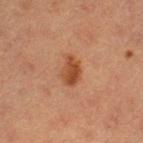<record>
<biopsy_status>not biopsied; imaged during a skin examination</biopsy_status>
<patient>
  <sex>female</sex>
  <age_approx>40</age_approx>
</patient>
<image>
  <source>total-body photography crop</source>
  <field_of_view_mm>15</field_of_view_mm>
</image>
<site>left thigh</site>
</record>